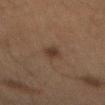Q: Was this lesion biopsied?
A: total-body-photography surveillance lesion; no biopsy
Q: How was this image acquired?
A: ~15 mm crop, total-body skin-cancer survey
Q: What is the anatomic site?
A: the mid back
Q: What lighting was used for the tile?
A: cross-polarized illumination
Q: Automated lesion metrics?
A: an area of roughly 3.5 mm²; a lesion color around L≈28 a*≈14 b*≈21 in CIELAB and a normalized lesion–skin contrast near 7.5; a border-irregularity rating of about 2.5/10, a color-variation rating of about 3/10, and a peripheral color-asymmetry measure near 1
Q: How large is the lesion?
A: about 2.5 mm
Q: Who is the patient?
A: male, aged around 65